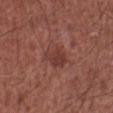Q: What are the patient's age and sex?
A: male, roughly 65 years of age
Q: What kind of image is this?
A: total-body-photography crop, ~15 mm field of view
Q: Illumination type?
A: white-light illumination
Q: Lesion location?
A: the left lower leg
Q: Automated lesion metrics?
A: a mean CIELAB color near L≈38 a*≈24 b*≈24
Q: What is the lesion's diameter?
A: about 3.5 mm